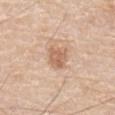* notes · no biopsy performed (imaged during a skin exam)
* acquisition · 15 mm crop, total-body photography
* illumination · white-light
* size · ~3 mm (longest diameter)
* subject · male, about 80 years old
* site · the mid back